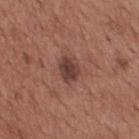Q: Where on the body is the lesion?
A: the back
Q: What lighting was used for the tile?
A: white-light
Q: How was this image acquired?
A: total-body-photography crop, ~15 mm field of view
Q: What are the patient's age and sex?
A: male, aged around 65